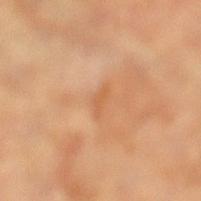{
  "biopsy_status": "not biopsied; imaged during a skin examination",
  "lighting": "cross-polarized",
  "image": {
    "source": "total-body photography crop",
    "field_of_view_mm": 15
  },
  "patient": {
    "sex": "female",
    "age_approx": 65
  },
  "site": "right leg",
  "automated_metrics": {
    "cielab_L": 58,
    "cielab_a": 24,
    "cielab_b": 39,
    "color_variation_0_10": 0.0,
    "peripheral_color_asymmetry": 0.0
  }
}